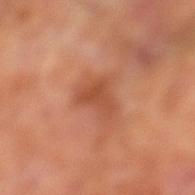Part of a total-body skin-imaging series; this lesion was reviewed on a skin check and was not flagged for biopsy. A male subject, in their 70s. The recorded lesion diameter is about 4 mm. From the left lower leg. A region of skin cropped from a whole-body photographic capture, roughly 15 mm wide. The total-body-photography lesion software estimated a lesion area of about 6.5 mm² and a symmetry-axis asymmetry near 0.45. The analysis additionally found a lesion color around L≈49 a*≈27 b*≈35 in CIELAB, about 8 CIELAB-L* units darker than the surrounding skin, and a normalized lesion–skin contrast near 6.5.